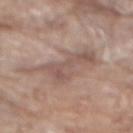The lesion was photographed on a routine skin check and not biopsied; there is no pathology result. A male patient approximately 80 years of age. The lesion is on the mid back. A roughly 15 mm field-of-view crop from a total-body skin photograph. The total-body-photography lesion software estimated peripheral color asymmetry of about 1.5.